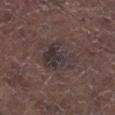Q: Was this lesion biopsied?
A: imaged on a skin check; not biopsied
Q: How large is the lesion?
A: ≈4.5 mm
Q: What did automated image analysis measure?
A: a footprint of about 11 mm², a shape eccentricity near 0.6, and a shape-asymmetry score of about 0.45 (0 = symmetric); a border-irregularity index near 6.5/10, a color-variation rating of about 4.5/10, and a peripheral color-asymmetry measure near 1.5
Q: What is the imaging modality?
A: ~15 mm tile from a whole-body skin photo
Q: Lesion location?
A: the right lower leg
Q: Who is the patient?
A: male, aged 68–72